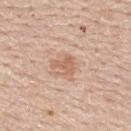Clinical impression: The lesion was tiled from a total-body skin photograph and was not biopsied. Image and clinical context: A 15 mm close-up tile from a total-body photography series done for melanoma screening. A male subject, approximately 60 years of age. The lesion is on the upper back.